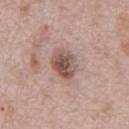Q: Was a biopsy performed?
A: catalogued during a skin exam; not biopsied
Q: What are the patient's age and sex?
A: male, aged around 70
Q: How large is the lesion?
A: ~3.5 mm (longest diameter)
Q: What is the anatomic site?
A: the chest
Q: How was this image acquired?
A: 15 mm crop, total-body photography
Q: How was the tile lit?
A: white-light illumination